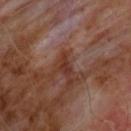Clinical impression:
The lesion was tiled from a total-body skin photograph and was not biopsied.
Context:
The lesion is on the chest. An algorithmic analysis of the crop reported an area of roughly 3 mm², a shape eccentricity near 0.85, and a shape-asymmetry score of about 0.5 (0 = symmetric). The software also gave an automated nevus-likeness rating near 0 out of 100 and a lesion-detection confidence of about 100/100. Approximately 3 mm at its widest. A male patient, aged 58 to 62. The tile uses cross-polarized illumination. A 15 mm close-up extracted from a 3D total-body photography capture.